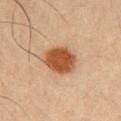Longest diameter approximately 4.5 mm. The tile uses cross-polarized illumination. From the chest. A male subject aged around 60. A 15 mm close-up extracted from a 3D total-body photography capture. An algorithmic analysis of the crop reported a lesion area of about 12 mm², an outline eccentricity of about 0.65 (0 = round, 1 = elongated), and a symmetry-axis asymmetry near 0.1. The analysis additionally found a lesion color around L≈43 a*≈20 b*≈32 in CIELAB and a normalized border contrast of about 11.5.